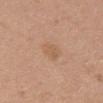No biopsy was performed on this lesion — it was imaged during a full skin examination and was not determined to be concerning.
The patient is a female about 40 years old.
A region of skin cropped from a whole-body photographic capture, roughly 15 mm wide.
The lesion is located on the front of the torso.
Automated tile analysis of the lesion measured a lesion area of about 4.5 mm², a shape eccentricity near 0.7, and a symmetry-axis asymmetry near 0.2. And it measured border irregularity of about 2 on a 0–10 scale, a within-lesion color-variation index near 2/10, and a peripheral color-asymmetry measure near 1. The software also gave a detector confidence of about 100 out of 100 that the crop contains a lesion.